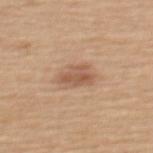Q: Was a biopsy performed?
A: total-body-photography surveillance lesion; no biopsy
Q: What is the anatomic site?
A: the upper back
Q: Illumination type?
A: white-light illumination
Q: Who is the patient?
A: female, aged around 55
Q: How was this image acquired?
A: ~15 mm crop, total-body skin-cancer survey
Q: Automated lesion metrics?
A: a classifier nevus-likeness of about 65/100 and lesion-presence confidence of about 100/100
Q: How large is the lesion?
A: ≈4 mm